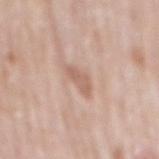Q: Was a biopsy performed?
A: no biopsy performed (imaged during a skin exam)
Q: What is the anatomic site?
A: the back
Q: What did automated image analysis measure?
A: a footprint of about 3.5 mm², an outline eccentricity of about 0.85 (0 = round, 1 = elongated), and a symmetry-axis asymmetry near 0.3; a lesion color around L≈61 a*≈20 b*≈28 in CIELAB, a lesion–skin lightness drop of about 9, and a normalized border contrast of about 6; a border-irregularity rating of about 3.5/10, a color-variation rating of about 1/10, and radial color variation of about 0.5
Q: How was this image acquired?
A: total-body-photography crop, ~15 mm field of view
Q: What lighting was used for the tile?
A: white-light illumination
Q: What are the patient's age and sex?
A: male, approximately 80 years of age
Q: How large is the lesion?
A: ~3 mm (longest diameter)